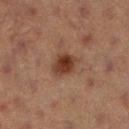workup: imaged on a skin check; not biopsied | TBP lesion metrics: a lesion area of about 7 mm², a shape eccentricity near 0.65, and a shape-asymmetry score of about 0.2 (0 = symmetric) | tile lighting: cross-polarized | lesion size: ≈3.5 mm | acquisition: ~15 mm crop, total-body skin-cancer survey | location: the right lower leg | subject: female, aged around 40.